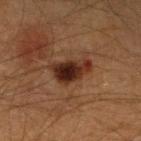Clinical impression:
The lesion was photographed on a routine skin check and not biopsied; there is no pathology result.
Image and clinical context:
Located on the left lower leg. A close-up tile cropped from a whole-body skin photograph, about 15 mm across. A male patient, aged around 50.